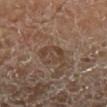Assessment:
This lesion was catalogued during total-body skin photography and was not selected for biopsy.
Background:
Imaged with cross-polarized lighting. A 15 mm close-up extracted from a 3D total-body photography capture. From the left lower leg. The patient is a male about 55 years old. About 3.5 mm across.